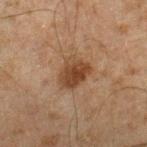The lesion was tiled from a total-body skin photograph and was not biopsied.
A male patient, aged approximately 45.
A roughly 15 mm field-of-view crop from a total-body skin photograph.
From the right lower leg.
Approximately 4 mm at its widest.
The tile uses cross-polarized illumination.
Automated image analysis of the tile measured a footprint of about 9.5 mm², an eccentricity of roughly 0.6, and a symmetry-axis asymmetry near 0.25. And it measured a classifier nevus-likeness of about 90/100 and a lesion-detection confidence of about 100/100.